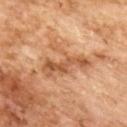Impression:
Imaged during a routine full-body skin examination; the lesion was not biopsied and no histopathology is available.
Clinical summary:
The lesion is on the upper back. A close-up tile cropped from a whole-body skin photograph, about 15 mm across. The patient is a female aged 58–62.